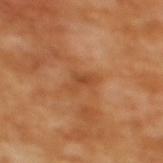  biopsy_status: not biopsied; imaged during a skin examination
  lighting: cross-polarized
  automated_metrics:
    area_mm2_approx: 4.5
    eccentricity: 0.85
    shape_asymmetry: 0.45
    cielab_L: 49
    cielab_a: 26
    cielab_b: 39
    vs_skin_darker_L: 8.0
    nevus_likeness_0_100: 0
    lesion_detection_confidence_0_100: 100
  patient:
    sex: female
    age_approx: 55
  site: upper back
  image:
    source: total-body photography crop
    field_of_view_mm: 15